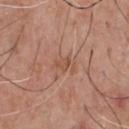Imaged during a routine full-body skin examination; the lesion was not biopsied and no histopathology is available.
The tile uses white-light illumination.
A roughly 15 mm field-of-view crop from a total-body skin photograph.
Measured at roughly 2.5 mm in maximum diameter.
The lesion is on the chest.
The lesion-visualizer software estimated a mean CIELAB color near L≈52 a*≈22 b*≈31, roughly 7 lightness units darker than nearby skin, and a normalized border contrast of about 5.5. And it measured lesion-presence confidence of about 100/100.
A male patient roughly 70 years of age.